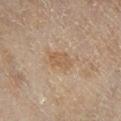| field | value |
|---|---|
| follow-up | total-body-photography surveillance lesion; no biopsy |
| patient | female, roughly 60 years of age |
| anatomic site | the right lower leg |
| acquisition | 15 mm crop, total-body photography |